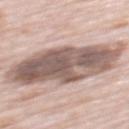workup: catalogued during a skin exam; not biopsied
tile lighting: white-light
diameter: ~11.5 mm (longest diameter)
body site: the mid back
acquisition: 15 mm crop, total-body photography
patient: male, about 80 years old